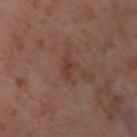notes — imaged on a skin check; not biopsied | patient — female, in their mid- to late 50s | lesion size — ≈2.5 mm | location — the right thigh | image-analysis metrics — an area of roughly 2.5 mm², a shape eccentricity near 0.85, and two-axis asymmetry of about 0.4 | image source — 15 mm crop, total-body photography | illumination — cross-polarized.